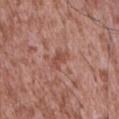Imaged during a routine full-body skin examination; the lesion was not biopsied and no histopathology is available. On the abdomen. A lesion tile, about 15 mm wide, cut from a 3D total-body photograph. A male patient aged around 45.